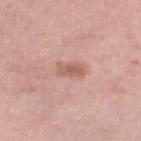Assessment: Captured during whole-body skin photography for melanoma surveillance; the lesion was not biopsied. Acquisition and patient details: Located on the right thigh. A female patient approximately 40 years of age. A 15 mm crop from a total-body photograph taken for skin-cancer surveillance. Automated tile analysis of the lesion measured an eccentricity of roughly 0.8 and two-axis asymmetry of about 0.25. About 3 mm across.